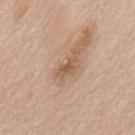Clinical impression: Captured during whole-body skin photography for melanoma surveillance; the lesion was not biopsied. Image and clinical context: The lesion-visualizer software estimated a footprint of about 3.5 mm², an outline eccentricity of about 0.85 (0 = round, 1 = elongated), and a shape-asymmetry score of about 0.3 (0 = symmetric). Cropped from a whole-body photographic skin survey; the tile spans about 15 mm. The lesion is on the mid back. Imaged with white-light lighting. A male patient, aged 63–67.